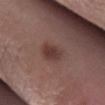Notes:
• biopsy status · catalogued during a skin exam; not biopsied
• lesion diameter · ~3.5 mm (longest diameter)
• location · the right lower leg
• image-analysis metrics · a footprint of about 5.5 mm², an eccentricity of roughly 0.8, and a shape-asymmetry score of about 0.25 (0 = symmetric); a mean CIELAB color near L≈37 a*≈20 b*≈21 and a lesion–skin lightness drop of about 9
• acquisition · 15 mm crop, total-body photography
• patient · male, aged around 45